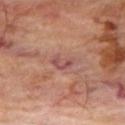Part of a total-body skin-imaging series; this lesion was reviewed on a skin check and was not flagged for biopsy.
Captured under cross-polarized illumination.
A male subject aged around 70.
Located on the right thigh.
A 15 mm crop from a total-body photograph taken for skin-cancer surveillance.